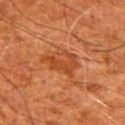anatomic site = the right upper arm; illumination = cross-polarized; patient = male, in their 80s; imaging modality = total-body-photography crop, ~15 mm field of view.